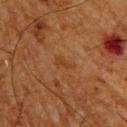notes = no biopsy performed (imaged during a skin exam)
lesion size = ~2.5 mm (longest diameter)
automated lesion analysis = a border-irregularity rating of about 4.5/10, a color-variation rating of about 0.5/10, and radial color variation of about 0
anatomic site = the upper back
tile lighting = cross-polarized
imaging modality = total-body-photography crop, ~15 mm field of view
subject = male, aged 63–67